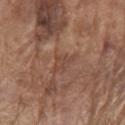* notes — catalogued during a skin exam; not biopsied
* imaging modality — ~15 mm crop, total-body skin-cancer survey
* patient — male, approximately 80 years of age
* body site — the left upper arm